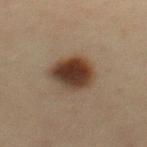Impression: Part of a total-body skin-imaging series; this lesion was reviewed on a skin check and was not flagged for biopsy. Clinical summary: On the mid back. Automated tile analysis of the lesion measured a lesion color around L≈34 a*≈15 b*≈24 in CIELAB and a lesion-to-skin contrast of about 13 (normalized; higher = more distinct). The analysis additionally found a color-variation rating of about 7/10 and peripheral color asymmetry of about 2. This is a cross-polarized tile. Measured at roughly 4.5 mm in maximum diameter. The subject is a male aged 48 to 52. Cropped from a total-body skin-imaging series; the visible field is about 15 mm.